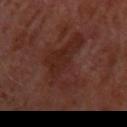A lesion tile, about 15 mm wide, cut from a 3D total-body photograph. A female subject, aged approximately 40. Longest diameter approximately 8 mm. The tile uses cross-polarized illumination. The lesion is on the left upper arm.